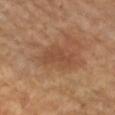Impression:
Part of a total-body skin-imaging series; this lesion was reviewed on a skin check and was not flagged for biopsy.
Context:
On the right upper arm. Captured under cross-polarized illumination. A female subject roughly 70 years of age. Cropped from a whole-body photographic skin survey; the tile spans about 15 mm. An algorithmic analysis of the crop reported an average lesion color of about L≈44 a*≈21 b*≈30 (CIELAB) and about 6 CIELAB-L* units darker than the surrounding skin. The analysis additionally found lesion-presence confidence of about 100/100.